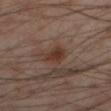– lesion size — ~3 mm (longest diameter)
– patient — male, aged around 55
– location — the right thigh
– automated metrics — a normalized border contrast of about 8
– imaging modality — 15 mm crop, total-body photography